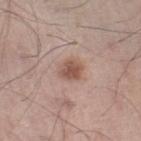Assessment: The lesion was tiled from a total-body skin photograph and was not biopsied. Image and clinical context: A roughly 15 mm field-of-view crop from a total-body skin photograph. From the right lower leg. The lesion-visualizer software estimated a nevus-likeness score of about 90/100 and a detector confidence of about 100 out of 100 that the crop contains a lesion. The subject is a male approximately 55 years of age.